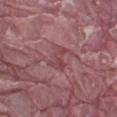{
  "biopsy_status": "not biopsied; imaged during a skin examination",
  "image": {
    "source": "total-body photography crop",
    "field_of_view_mm": 15
  },
  "site": "leg",
  "patient": {
    "sex": "male",
    "age_approx": 40
  }
}